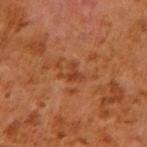{"biopsy_status": "not biopsied; imaged during a skin examination", "automated_metrics": {"area_mm2_approx": 4.0, "eccentricity": 0.55, "shape_asymmetry": 0.6, "cielab_L": 40, "cielab_a": 26, "cielab_b": 35, "vs_skin_darker_L": 7.0, "vs_skin_contrast_norm": 6.0, "border_irregularity_0_10": 6.5, "color_variation_0_10": 0.5, "peripheral_color_asymmetry": 0.0, "nevus_likeness_0_100": 0}, "lesion_size": {"long_diameter_mm_approx": 2.5}, "lighting": "cross-polarized", "image": {"source": "total-body photography crop", "field_of_view_mm": 15}, "patient": {"sex": "male", "age_approx": 60}, "site": "right upper arm"}